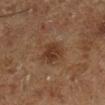Q: Is there a histopathology result?
A: no biopsy performed (imaged during a skin exam)
Q: Lesion location?
A: the right lower leg
Q: Who is the patient?
A: male, in their mid- to late 70s
Q: Automated lesion metrics?
A: a footprint of about 8 mm² and an outline eccentricity of about 0.45 (0 = round, 1 = elongated); an automated nevus-likeness rating near 65 out of 100 and a lesion-detection confidence of about 100/100
Q: How was this image acquired?
A: total-body-photography crop, ~15 mm field of view
Q: How was the tile lit?
A: cross-polarized illumination